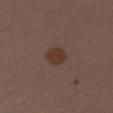Notes:
* biopsy status — imaged on a skin check; not biopsied
* automated metrics — border irregularity of about 1.5 on a 0–10 scale, a within-lesion color-variation index near 1.5/10, and radial color variation of about 0.5; a classifier nevus-likeness of about 95/100
* patient — male, aged approximately 40
* image source — total-body-photography crop, ~15 mm field of view
* diameter — ≈2.5 mm
* anatomic site — the right upper arm